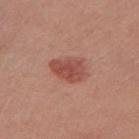Assessment: The lesion was tiled from a total-body skin photograph and was not biopsied. Background: Cropped from a whole-body photographic skin survey; the tile spans about 15 mm. The lesion-visualizer software estimated a lesion color around L≈50 a*≈27 b*≈27 in CIELAB, roughly 10 lightness units darker than nearby skin, and a lesion-to-skin contrast of about 7.5 (normalized; higher = more distinct). The analysis additionally found border irregularity of about 2.5 on a 0–10 scale and internal color variation of about 3 on a 0–10 scale. From the front of the torso. The subject is a female aged around 65.